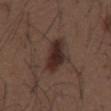lighting: white-light
patient:
  sex: male
  age_approx: 50
lesion_size:
  long_diameter_mm_approx: 4.5
image:
  source: total-body photography crop
  field_of_view_mm: 15
site: mid back